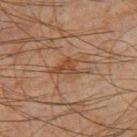| feature | finding |
|---|---|
| biopsy status | no biopsy performed (imaged during a skin exam) |
| acquisition | ~15 mm crop, total-body skin-cancer survey |
| location | the right thigh |
| patient | male, aged 63–67 |